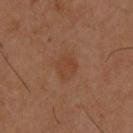<case>
<automated_metrics>
  <nevus_likeness_0_100>5</nevus_likeness_0_100>
  <lesion_detection_confidence_0_100>100</lesion_detection_confidence_0_100>
</automated_metrics>
<image>
  <source>total-body photography crop</source>
  <field_of_view_mm>15</field_of_view_mm>
</image>
<lighting>cross-polarized</lighting>
<site>upper back</site>
<patient>
  <sex>male</sex>
  <age_approx>40</age_approx>
</patient>
</case>